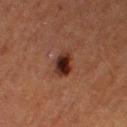Q: Was this lesion biopsied?
A: total-body-photography surveillance lesion; no biopsy
Q: What is the lesion's diameter?
A: ≈3.5 mm
Q: Patient demographics?
A: female, aged approximately 40
Q: What kind of image is this?
A: total-body-photography crop, ~15 mm field of view
Q: What did automated image analysis measure?
A: a lesion area of about 6.5 mm² and two-axis asymmetry of about 0.2; a lesion color around L≈24 a*≈20 b*≈22 in CIELAB, a lesion–skin lightness drop of about 12, and a lesion-to-skin contrast of about 12.5 (normalized; higher = more distinct); a classifier nevus-likeness of about 95/100
Q: Where on the body is the lesion?
A: the right thigh
Q: What lighting was used for the tile?
A: cross-polarized illumination